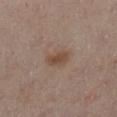Captured during whole-body skin photography for melanoma surveillance; the lesion was not biopsied. Imaged with cross-polarized lighting. Approximately 3 mm at its widest. Located on the left lower leg. The subject is a female in their mid-50s. An algorithmic analysis of the crop reported a lesion area of about 4.5 mm² and an outline eccentricity of about 0.7 (0 = round, 1 = elongated). And it measured a nevus-likeness score of about 80/100 and a lesion-detection confidence of about 100/100. A region of skin cropped from a whole-body photographic capture, roughly 15 mm wide.